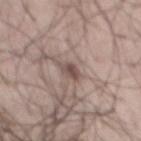notes = catalogued during a skin exam; not biopsied | subject = male, approximately 50 years of age | anatomic site = the mid back | image = total-body-photography crop, ~15 mm field of view.